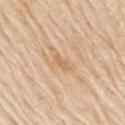Recorded during total-body skin imaging; not selected for excision or biopsy.
The patient is a male in their 80s.
This is a white-light tile.
The recorded lesion diameter is about 3 mm.
A close-up tile cropped from a whole-body skin photograph, about 15 mm across.
Located on the right upper arm.